Approximately 15.5 mm at its widest · the subject is a female aged approximately 70 · a 15 mm close-up extracted from a 3D total-body photography capture · captured under cross-polarized illumination · the lesion is on the front of the torso — 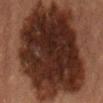Conclusion: Histopathological examination showed a dysplastic (Clark) nevus — a benign skin lesion.